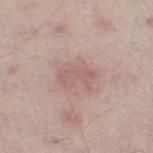Assessment: This lesion was catalogued during total-body skin photography and was not selected for biopsy. Clinical summary: A lesion tile, about 15 mm wide, cut from a 3D total-body photograph. The lesion is on the leg. A male subject aged approximately 65.